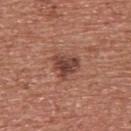biopsy status=catalogued during a skin exam; not biopsied
diameter=~3.5 mm (longest diameter)
image=~15 mm tile from a whole-body skin photo
tile lighting=white-light illumination
anatomic site=the chest
patient=male, aged around 45